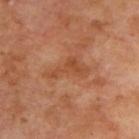Notes:
– patient · male, in their 70s
– image · total-body-photography crop, ~15 mm field of view
– tile lighting · cross-polarized illumination
– body site · the upper back
– diameter · ~5 mm (longest diameter)